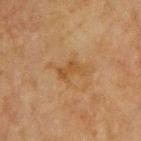TBP lesion metrics: a footprint of about 6.5 mm² and an outline eccentricity of about 0.8 (0 = round, 1 = elongated); an average lesion color of about L≈41 a*≈16 b*≈33 (CIELAB), roughly 5 lightness units darker than nearby skin, and a normalized border contrast of about 6; a nevus-likeness score of about 0/100 and a lesion-detection confidence of about 100/100 | diameter: ≈4 mm | anatomic site: the chest | patient: male, in their mid-60s | imaging modality: ~15 mm crop, total-body skin-cancer survey.